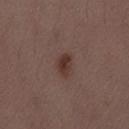Captured during whole-body skin photography for melanoma surveillance; the lesion was not biopsied.
The subject is a male aged 28 to 32.
The recorded lesion diameter is about 3 mm.
This is a white-light tile.
The total-body-photography lesion software estimated a mean CIELAB color near L≈34 a*≈18 b*≈21, a lesion–skin lightness drop of about 9, and a normalized lesion–skin contrast near 8. The analysis additionally found a border-irregularity rating of about 2.5/10.
This image is a 15 mm lesion crop taken from a total-body photograph.
Located on the lower back.